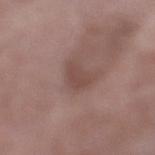- subject — female, in their 70s
- anatomic site — the left lower leg
- image — total-body-photography crop, ~15 mm field of view
- lesion diameter — ≈3.5 mm
- automated metrics — a mean CIELAB color near L≈46 a*≈19 b*≈22, a lesion–skin lightness drop of about 7, and a lesion-to-skin contrast of about 5.5 (normalized; higher = more distinct); lesion-presence confidence of about 90/100
- tile lighting — white-light illumination